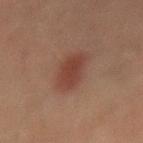Part of a total-body skin-imaging series; this lesion was reviewed on a skin check and was not flagged for biopsy.
A roughly 15 mm field-of-view crop from a total-body skin photograph.
The total-body-photography lesion software estimated a lesion area of about 9.5 mm², an eccentricity of roughly 0.85, and a symmetry-axis asymmetry near 0.15. The analysis additionally found an average lesion color of about L≈33 a*≈19 b*≈22 (CIELAB) and a lesion-to-skin contrast of about 7.5 (normalized; higher = more distinct). And it measured a classifier nevus-likeness of about 100/100 and a lesion-detection confidence of about 100/100.
Located on the mid back.
A male patient aged 43–47.
Measured at roughly 4.5 mm in maximum diameter.